The subject is a female aged around 70. Located on the head or neck. A 15 mm crop from a total-body photograph taken for skin-cancer surveillance:
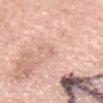Findings:
– image-analysis metrics · a footprint of about 1 mm² and an eccentricity of roughly 0.8; an average lesion color of about L≈67 a*≈21 b*≈29 (CIELAB), about 6 CIELAB-L* units darker than the surrounding skin, and a normalized lesion–skin contrast near 3.5; a nevus-likeness score of about 0/100 and a lesion-detection confidence of about 100/100
– diameter · ≈1.5 mm
– diagnosis · a solar lentigo — a benign skin lesion